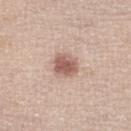The lesion was tiled from a total-body skin photograph and was not biopsied. Imaged with white-light lighting. The subject is a male about 65 years old. This image is a 15 mm lesion crop taken from a total-body photograph. An algorithmic analysis of the crop reported roughly 13 lightness units darker than nearby skin and a normalized border contrast of about 8.5. And it measured a border-irregularity rating of about 2/10, internal color variation of about 3 on a 0–10 scale, and peripheral color asymmetry of about 1. It also reported a nevus-likeness score of about 85/100 and a lesion-detection confidence of about 100/100. Located on the left lower leg. About 3 mm across.